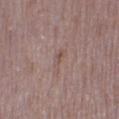<case>
  <site>left thigh</site>
  <lighting>white-light</lighting>
  <automated_metrics>
    <area_mm2_approx>3.5</area_mm2_approx>
    <shape_asymmetry>0.5</shape_asymmetry>
    <cielab_L>52</cielab_L>
    <cielab_a>17</cielab_a>
    <cielab_b>22</cielab_b>
    <vs_skin_darker_L>5.0</vs_skin_darker_L>
    <vs_skin_contrast_norm>4.5</vs_skin_contrast_norm>
    <nevus_likeness_0_100>0</nevus_likeness_0_100>
    <lesion_detection_confidence_0_100>100</lesion_detection_confidence_0_100>
  </automated_metrics>
  <lesion_size>
    <long_diameter_mm_approx>3.0</long_diameter_mm_approx>
  </lesion_size>
  <image>
    <source>total-body photography crop</source>
    <field_of_view_mm>15</field_of_view_mm>
  </image>
  <patient>
    <sex>female</sex>
    <age_approx>30</age_approx>
  </patient>
</case>